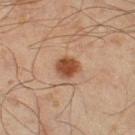No biopsy was performed on this lesion — it was imaged during a full skin examination and was not determined to be concerning. Captured under cross-polarized illumination. The lesion is located on the right thigh. A male patient, in their mid- to late 40s. A region of skin cropped from a whole-body photographic capture, roughly 15 mm wide. The lesion-visualizer software estimated an eccentricity of roughly 0.3 and a shape-asymmetry score of about 0.15 (0 = symmetric). The software also gave a mean CIELAB color near L≈38 a*≈19 b*≈28 and a normalized border contrast of about 10. The analysis additionally found a border-irregularity rating of about 1.5/10, a color-variation rating of about 2.5/10, and a peripheral color-asymmetry measure near 1.